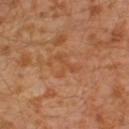follow-up: total-body-photography surveillance lesion; no biopsy | patient: male, aged around 30 | automated lesion analysis: border irregularity of about 7.5 on a 0–10 scale, internal color variation of about 0 on a 0–10 scale, and a peripheral color-asymmetry measure near 0; a classifier nevus-likeness of about 0/100 and a lesion-detection confidence of about 100/100 | illumination: cross-polarized illumination | location: the leg | image: ~15 mm crop, total-body skin-cancer survey | size: about 3.5 mm.